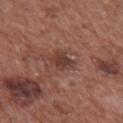{
  "biopsy_status": "not biopsied; imaged during a skin examination",
  "image": {
    "source": "total-body photography crop",
    "field_of_view_mm": 15
  },
  "lighting": "white-light",
  "lesion_size": {
    "long_diameter_mm_approx": 3.0
  },
  "site": "front of the torso",
  "patient": {
    "sex": "male",
    "age_approx": 75
  },
  "automated_metrics": {
    "lesion_detection_confidence_0_100": 100
  }
}